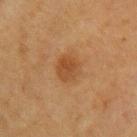Impression:
The lesion was photographed on a routine skin check and not biopsied; there is no pathology result.
Context:
The lesion is located on the left upper arm. A female subject roughly 55 years of age. A 15 mm crop from a total-body photograph taken for skin-cancer surveillance.